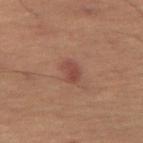notes: imaged on a skin check; not biopsied
imaging modality: ~15 mm crop, total-body skin-cancer survey
illumination: cross-polarized illumination
automated lesion analysis: a lesion area of about 4.5 mm², an eccentricity of roughly 0.85, and a shape-asymmetry score of about 0.2 (0 = symmetric); a nevus-likeness score of about 60/100 and lesion-presence confidence of about 100/100
site: the right thigh
lesion diameter: ~3.5 mm (longest diameter)
subject: female, in their 80s